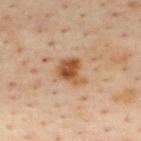Impression: No biopsy was performed on this lesion — it was imaged during a full skin examination and was not determined to be concerning. Clinical summary: Approximately 3.5 mm at its widest. A lesion tile, about 15 mm wide, cut from a 3D total-body photograph. The total-body-photography lesion software estimated a footprint of about 7 mm², a shape eccentricity near 0.6, and a symmetry-axis asymmetry near 0.3. It also reported a border-irregularity rating of about 3/10 and peripheral color asymmetry of about 1.5. Located on the upper back. A male patient, in their mid- to late 40s. The tile uses cross-polarized illumination.